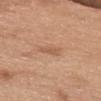Q: Is there a histopathology result?
A: catalogued during a skin exam; not biopsied
Q: How was the tile lit?
A: white-light
Q: How was this image acquired?
A: total-body-photography crop, ~15 mm field of view
Q: What did automated image analysis measure?
A: a lesion area of about 3.5 mm², an eccentricity of roughly 0.8, and a symmetry-axis asymmetry near 0.4; an average lesion color of about L≈57 a*≈21 b*≈33 (CIELAB) and a lesion-to-skin contrast of about 5 (normalized; higher = more distinct); a classifier nevus-likeness of about 0/100 and lesion-presence confidence of about 100/100
Q: Who is the patient?
A: female, roughly 65 years of age
Q: Lesion size?
A: ~2.5 mm (longest diameter)
Q: Where on the body is the lesion?
A: the chest